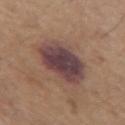<lesion>
<biopsy_status>not biopsied; imaged during a skin examination</biopsy_status>
<patient>
  <sex>male</sex>
  <age_approx>65</age_approx>
</patient>
<lesion_size>
  <long_diameter_mm_approx>5.5</long_diameter_mm_approx>
</lesion_size>
<image>
  <source>total-body photography crop</source>
  <field_of_view_mm>15</field_of_view_mm>
</image>
<site>arm</site>
<lighting>white-light</lighting>
</lesion>